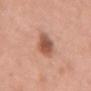Recorded during total-body skin imaging; not selected for excision or biopsy. From the upper back. A region of skin cropped from a whole-body photographic capture, roughly 15 mm wide. A female subject, roughly 70 years of age.